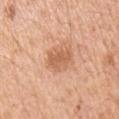Clinical impression: Recorded during total-body skin imaging; not selected for excision or biopsy. Background: An algorithmic analysis of the crop reported a footprint of about 7 mm², an outline eccentricity of about 0.6 (0 = round, 1 = elongated), and two-axis asymmetry of about 0.3. It also reported about 10 CIELAB-L* units darker than the surrounding skin and a normalized border contrast of about 6.5. A 15 mm close-up tile from a total-body photography series done for melanoma screening. Located on the right upper arm. A male subject aged around 55. Longest diameter approximately 3.5 mm.